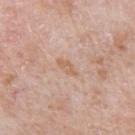The lesion was tiled from a total-body skin photograph and was not biopsied.
A 15 mm crop from a total-body photograph taken for skin-cancer surveillance.
A male patient aged around 80.
The recorded lesion diameter is about 2.5 mm.
From the right upper arm.
The tile uses white-light illumination.